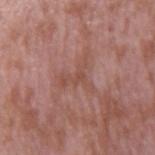Clinical impression:
This lesion was catalogued during total-body skin photography and was not selected for biopsy.
Clinical summary:
A close-up tile cropped from a whole-body skin photograph, about 15 mm across. A male subject aged 38 to 42. The total-body-photography lesion software estimated a normalized lesion–skin contrast near 5. The analysis additionally found a color-variation rating of about 1/10 and peripheral color asymmetry of about 0. The analysis additionally found a nevus-likeness score of about 0/100. Captured under white-light illumination. The lesion is on the right upper arm.